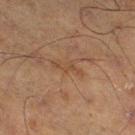location: the right thigh | acquisition: ~15 mm tile from a whole-body skin photo | diameter: about 3.5 mm | patient: male, aged 68 to 72.